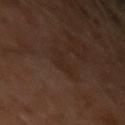A male patient approximately 30 years of age.
This is a cross-polarized tile.
A roughly 15 mm field-of-view crop from a total-body skin photograph.
Automated tile analysis of the lesion measured a mean CIELAB color near L≈23 a*≈14 b*≈22, roughly 3 lightness units darker than nearby skin, and a normalized border contrast of about 4.5. The analysis additionally found a border-irregularity index near 6.5/10, internal color variation of about 0.5 on a 0–10 scale, and a peripheral color-asymmetry measure near 0. The analysis additionally found a nevus-likeness score of about 0/100 and lesion-presence confidence of about 95/100.
The lesion is on the left forearm.
About 3 mm across.